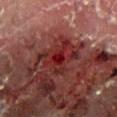The lesion was tiled from a total-body skin photograph and was not biopsied. A male patient aged approximately 55. The recorded lesion diameter is about 4.5 mm. The total-body-photography lesion software estimated a lesion area of about 13 mm² and a symmetry-axis asymmetry near 0.45. The software also gave border irregularity of about 6 on a 0–10 scale, a color-variation rating of about 7.5/10, and radial color variation of about 2. The analysis additionally found a nevus-likeness score of about 0/100 and a lesion-detection confidence of about 80/100. The lesion is located on the leg. A lesion tile, about 15 mm wide, cut from a 3D total-body photograph.